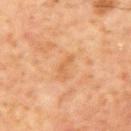- biopsy status: imaged on a skin check; not biopsied